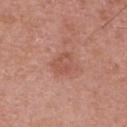Q: Was a biopsy performed?
A: catalogued during a skin exam; not biopsied
Q: What kind of image is this?
A: 15 mm crop, total-body photography
Q: Who is the patient?
A: male, about 50 years old
Q: What lighting was used for the tile?
A: white-light illumination
Q: Lesion size?
A: ~2.5 mm (longest diameter)
Q: Automated lesion metrics?
A: a lesion area of about 4.5 mm²; border irregularity of about 2.5 on a 0–10 scale, internal color variation of about 2.5 on a 0–10 scale, and radial color variation of about 0.5
Q: What is the anatomic site?
A: the arm